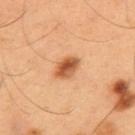workup: imaged on a skin check; not biopsied | lighting: cross-polarized | body site: the upper back | automated lesion analysis: an area of roughly 6 mm² and a shape-asymmetry score of about 0.15 (0 = symmetric); a border-irregularity index near 1.5/10 and a within-lesion color-variation index near 6/10 | imaging modality: ~15 mm crop, total-body skin-cancer survey | subject: male, approximately 55 years of age | lesion diameter: ≈3.5 mm.